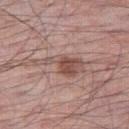{
  "biopsy_status": "not biopsied; imaged during a skin examination",
  "lesion_size": {
    "long_diameter_mm_approx": 6.0
  },
  "image": {
    "source": "total-body photography crop",
    "field_of_view_mm": 15
  },
  "site": "right thigh",
  "lighting": "white-light",
  "patient": {
    "sex": "male",
    "age_approx": 55
  }
}